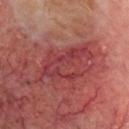Notes:
• notes: imaged on a skin check; not biopsied
• TBP lesion metrics: a mean CIELAB color near L≈39 a*≈32 b*≈22 and a lesion-to-skin contrast of about 6 (normalized; higher = more distinct); lesion-presence confidence of about 75/100
• site: the head or neck
• subject: male, aged 63–67
• imaging modality: ~15 mm tile from a whole-body skin photo
• diameter: ≈7 mm